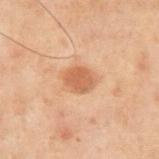Clinical impression: The lesion was photographed on a routine skin check and not biopsied; there is no pathology result. Acquisition and patient details: A male subject, about 70 years old. The lesion is on the front of the torso. A lesion tile, about 15 mm wide, cut from a 3D total-body photograph. Captured under cross-polarized illumination.